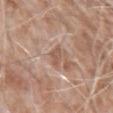Findings:
• body site — the arm
• subject — male, roughly 75 years of age
• imaging modality — total-body-photography crop, ~15 mm field of view